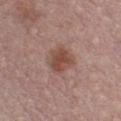Impression:
Part of a total-body skin-imaging series; this lesion was reviewed on a skin check and was not flagged for biopsy.
Context:
A 15 mm crop from a total-body photograph taken for skin-cancer surveillance. The tile uses white-light illumination. Measured at roughly 3.5 mm in maximum diameter. The subject is a male aged 28–32. Automated image analysis of the tile measured a shape eccentricity near 0.4 and a shape-asymmetry score of about 0.2 (0 = symmetric). The analysis additionally found a lesion color around L≈48 a*≈21 b*≈25 in CIELAB, about 10 CIELAB-L* units darker than the surrounding skin, and a normalized lesion–skin contrast near 7.5. The analysis additionally found a border-irregularity index near 2/10, a color-variation rating of about 3/10, and a peripheral color-asymmetry measure near 1. And it measured a nevus-likeness score of about 65/100.